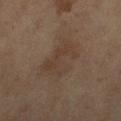- notes · catalogued during a skin exam; not biopsied
- image-analysis metrics · a lesion area of about 15 mm², a shape eccentricity near 0.75, and a symmetry-axis asymmetry near 0.35; a mean CIELAB color near L≈33 a*≈12 b*≈22, a lesion–skin lightness drop of about 5, and a normalized border contrast of about 5; an automated nevus-likeness rating near 0 out of 100 and a lesion-detection confidence of about 100/100
- location · the leg
- subject · female, approximately 60 years of age
- lesion size · about 5 mm
- acquisition · 15 mm crop, total-body photography